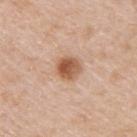{"patient": {"sex": "male", "age_approx": 50}, "site": "back", "image": {"source": "total-body photography crop", "field_of_view_mm": 15}}